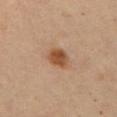workup = no biopsy performed (imaged during a skin exam)
subject = female, aged 43 to 47
size = ~3 mm (longest diameter)
lighting = cross-polarized illumination
automated lesion analysis = a classifier nevus-likeness of about 100/100
imaging modality = total-body-photography crop, ~15 mm field of view
location = the arm